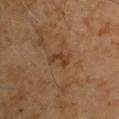Background:
Approximately 2.5 mm at its widest. A male subject, in their 60s. The lesion-visualizer software estimated a footprint of about 3 mm², an outline eccentricity of about 0.85 (0 = round, 1 = elongated), and two-axis asymmetry of about 0.45. The software also gave roughly 7 lightness units darker than nearby skin. It also reported a border-irregularity index near 5.5/10, internal color variation of about 0 on a 0–10 scale, and a peripheral color-asymmetry measure near 0. It also reported an automated nevus-likeness rating near 0 out of 100 and a lesion-detection confidence of about 100/100. Captured under cross-polarized illumination. A 15 mm close-up extracted from a 3D total-body photography capture. The lesion is located on the right arm.